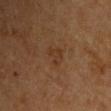follow-up: imaged on a skin check; not biopsied
lesion size: about 2.5 mm
body site: the back
automated lesion analysis: a lesion area of about 4 mm², an outline eccentricity of about 0.7 (0 = round, 1 = elongated), and two-axis asymmetry of about 0.45; an average lesion color of about L≈31 a*≈17 b*≈28 (CIELAB), about 5 CIELAB-L* units darker than the surrounding skin, and a normalized border contrast of about 5; border irregularity of about 4 on a 0–10 scale, internal color variation of about 2.5 on a 0–10 scale, and a peripheral color-asymmetry measure near 1; a lesion-detection confidence of about 100/100
illumination: cross-polarized illumination
image source: total-body-photography crop, ~15 mm field of view
subject: male, aged approximately 65